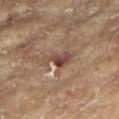Longest diameter approximately 3.5 mm. Cropped from a total-body skin-imaging series; the visible field is about 15 mm. Automated tile analysis of the lesion measured a lesion area of about 5 mm², an eccentricity of roughly 0.85, and two-axis asymmetry of about 0.4. The software also gave a peripheral color-asymmetry measure near 1.5. The analysis additionally found an automated nevus-likeness rating near 0 out of 100 and a detector confidence of about 90 out of 100 that the crop contains a lesion. The patient is a female in their 80s. The lesion is on the left arm.